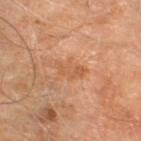Imaged during a routine full-body skin examination; the lesion was not biopsied and no histopathology is available.
The patient is a male approximately 70 years of age.
Cropped from a total-body skin-imaging series; the visible field is about 15 mm.
Longest diameter approximately 3.5 mm.
The lesion is located on the leg.
Imaged with cross-polarized lighting.
An algorithmic analysis of the crop reported an average lesion color of about L≈56 a*≈24 b*≈37 (CIELAB), about 6 CIELAB-L* units darker than the surrounding skin, and a normalized lesion–skin contrast near 5.5. And it measured an automated nevus-likeness rating near 0 out of 100 and a lesion-detection confidence of about 100/100.